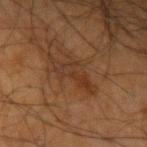workup — total-body-photography surveillance lesion; no biopsy
patient — male, in their mid-60s
body site — the left upper arm
tile lighting — cross-polarized
image — total-body-photography crop, ~15 mm field of view
size — about 6.5 mm